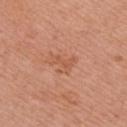biopsy status = no biopsy performed (imaged during a skin exam)
anatomic site = the right upper arm
subject = male, approximately 70 years of age
lesion size = ~3.5 mm (longest diameter)
acquisition = ~15 mm tile from a whole-body skin photo
lighting = white-light illumination